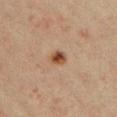{"biopsy_status": "not biopsied; imaged during a skin examination", "lighting": "cross-polarized", "image": {"source": "total-body photography crop", "field_of_view_mm": 15}, "automated_metrics": {"eccentricity": 0.5, "shape_asymmetry": 0.25, "cielab_L": 40, "cielab_a": 19, "cielab_b": 29, "vs_skin_darker_L": 12.0, "vs_skin_contrast_norm": 10.5, "border_irregularity_0_10": 2.0, "color_variation_0_10": 4.0, "peripheral_color_asymmetry": 1.5}, "lesion_size": {"long_diameter_mm_approx": 2.0}, "patient": {"sex": "female", "age_approx": 40}, "site": "chest"}